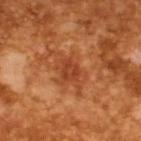Clinical impression:
The lesion was photographed on a routine skin check and not biopsied; there is no pathology result.
Context:
The patient is a male about 65 years old. Imaged with cross-polarized lighting. The lesion-visualizer software estimated a lesion area of about 4 mm², a shape eccentricity near 0.55, and a shape-asymmetry score of about 0.35 (0 = symmetric). And it measured an automated nevus-likeness rating near 0 out of 100 and lesion-presence confidence of about 100/100. A close-up tile cropped from a whole-body skin photograph, about 15 mm across. Measured at roughly 2.5 mm in maximum diameter.